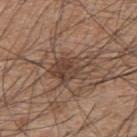notes: catalogued during a skin exam; not biopsied | location: the back | patient: male, in their mid- to late 40s | image source: ~15 mm tile from a whole-body skin photo.